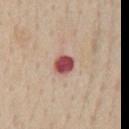{"biopsy_status": "not biopsied; imaged during a skin examination", "image": {"source": "total-body photography crop", "field_of_view_mm": 15}, "site": "chest", "lesion_size": {"long_diameter_mm_approx": 2.5}, "lighting": "white-light", "patient": {"sex": "male", "age_approx": 60}}